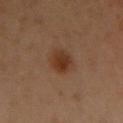Part of a total-body skin-imaging series; this lesion was reviewed on a skin check and was not flagged for biopsy. The recorded lesion diameter is about 3 mm. Cropped from a total-body skin-imaging series; the visible field is about 15 mm. Captured under cross-polarized illumination. A female patient, aged 58 to 62. On the chest.